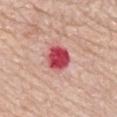No biopsy was performed on this lesion — it was imaged during a full skin examination and was not determined to be concerning. Captured under white-light illumination. Approximately 3.5 mm at its widest. The lesion-visualizer software estimated border irregularity of about 1.5 on a 0–10 scale, a within-lesion color-variation index near 3.5/10, and radial color variation of about 1. And it measured a classifier nevus-likeness of about 0/100. The lesion is on the mid back. A female subject, aged approximately 65. A close-up tile cropped from a whole-body skin photograph, about 15 mm across.